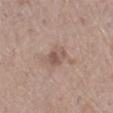Acquisition and patient details:
The lesion-visualizer software estimated an area of roughly 5 mm² and a symmetry-axis asymmetry near 0.2. The software also gave a lesion color around L≈54 a*≈17 b*≈24 in CIELAB, roughly 9 lightness units darker than nearby skin, and a normalized lesion–skin contrast near 6. And it measured border irregularity of about 2 on a 0–10 scale and a within-lesion color-variation index near 4.5/10. The analysis additionally found a nevus-likeness score of about 0/100 and a detector confidence of about 100 out of 100 that the crop contains a lesion. This is a white-light tile. A female subject in their mid- to late 50s. The recorded lesion diameter is about 3 mm. A region of skin cropped from a whole-body photographic capture, roughly 15 mm wide. From the right lower leg.